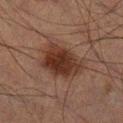Q: What are the patient's age and sex?
A: male, about 50 years old
Q: What is the imaging modality?
A: total-body-photography crop, ~15 mm field of view
Q: What is the anatomic site?
A: the right lower leg